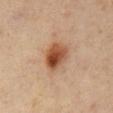A close-up tile cropped from a whole-body skin photograph, about 15 mm across.
From the abdomen.
Imaged with cross-polarized lighting.
The patient is a male aged 58–62.
Approximately 4 mm at its widest.